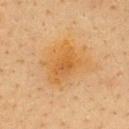| field | value |
|---|---|
| notes | total-body-photography surveillance lesion; no biopsy |
| size | about 5 mm |
| automated metrics | an area of roughly 16 mm² and a shape eccentricity near 0.6; a mean CIELAB color near L≈53 a*≈19 b*≈41, about 6 CIELAB-L* units darker than the surrounding skin, and a normalized lesion–skin contrast near 6.5; a classifier nevus-likeness of about 55/100 and lesion-presence confidence of about 100/100 |
| subject | female, aged 38 to 42 |
| anatomic site | the upper back |
| imaging modality | ~15 mm crop, total-body skin-cancer survey |